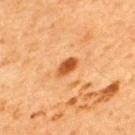Impression:
No biopsy was performed on this lesion — it was imaged during a full skin examination and was not determined to be concerning.
Context:
This is a cross-polarized tile. Cropped from a total-body skin-imaging series; the visible field is about 15 mm. A male patient in their mid-60s. The lesion is on the upper back.